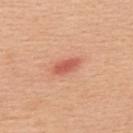- image-analysis metrics — a lesion color around L≈58 a*≈32 b*≈33 in CIELAB and roughly 12 lightness units darker than nearby skin; a color-variation rating of about 1/10; an automated nevus-likeness rating near 80 out of 100 and a detector confidence of about 100 out of 100 that the crop contains a lesion
- illumination — white-light
- body site — the upper back
- imaging modality — total-body-photography crop, ~15 mm field of view
- patient — male, aged around 55
- diameter — ~3.5 mm (longest diameter)